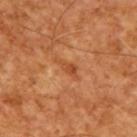Assessment:
Part of a total-body skin-imaging series; this lesion was reviewed on a skin check and was not flagged for biopsy.
Context:
The subject is a male in their mid- to late 60s. A roughly 15 mm field-of-view crop from a total-body skin photograph.